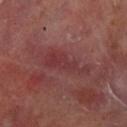| feature | finding |
|---|---|
| biopsy status | catalogued during a skin exam; not biopsied |
| body site | the left lower leg |
| subject | male, aged approximately 70 |
| tile lighting | cross-polarized illumination |
| imaging modality | 15 mm crop, total-body photography |